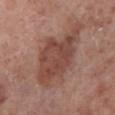Q: Was this lesion biopsied?
A: imaged on a skin check; not biopsied
Q: What kind of image is this?
A: ~15 mm tile from a whole-body skin photo
Q: Patient demographics?
A: male, in their 70s
Q: What is the anatomic site?
A: the leg